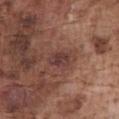Background:
A male patient, in their mid- to late 70s. The tile uses white-light illumination. A region of skin cropped from a whole-body photographic capture, roughly 15 mm wide. The lesion is located on the front of the torso.